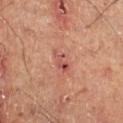<lesion>
  <biopsy_status>not biopsied; imaged during a skin examination</biopsy_status>
  <patient>
    <sex>male</sex>
    <age_approx>50</age_approx>
  </patient>
  <lesion_size>
    <long_diameter_mm_approx>3.0</long_diameter_mm_approx>
  </lesion_size>
  <lighting>cross-polarized</lighting>
  <image>
    <source>total-body photography crop</source>
    <field_of_view_mm>15</field_of_view_mm>
  </image>
  <site>right lower leg</site>
</lesion>